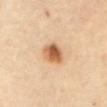Clinical summary:
This image is a 15 mm lesion crop taken from a total-body photograph. The lesion's longest dimension is about 3.5 mm. The lesion-visualizer software estimated a lesion area of about 7 mm², an eccentricity of roughly 0.6, and a symmetry-axis asymmetry near 0.2. And it measured a lesion–skin lightness drop of about 15 and a normalized border contrast of about 9.5. And it measured lesion-presence confidence of about 100/100. A female patient, about 60 years old. From the abdomen.